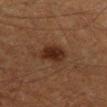No biopsy was performed on this lesion — it was imaged during a full skin examination and was not determined to be concerning.
A close-up tile cropped from a whole-body skin photograph, about 15 mm across.
Imaged with cross-polarized lighting.
The recorded lesion diameter is about 3.5 mm.
The lesion-visualizer software estimated a within-lesion color-variation index near 2.5/10 and a peripheral color-asymmetry measure near 0.5. The software also gave a classifier nevus-likeness of about 95/100.
A male patient approximately 60 years of age.
On the left lower leg.